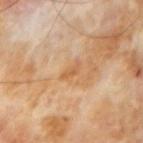The lesion was photographed on a routine skin check and not biopsied; there is no pathology result.
A 15 mm crop from a total-body photograph taken for skin-cancer surveillance.
Automated tile analysis of the lesion measured an outline eccentricity of about 0.95 (0 = round, 1 = elongated) and two-axis asymmetry of about 0.45. And it measured an automated nevus-likeness rating near 0 out of 100 and a lesion-detection confidence of about 100/100.
The subject is a male aged around 70.
The lesion is on the left forearm.
Approximately 3 mm at its widest.